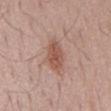{
  "biopsy_status": "not biopsied; imaged during a skin examination",
  "image": {
    "source": "total-body photography crop",
    "field_of_view_mm": 15
  },
  "site": "chest",
  "patient": {
    "sex": "male",
    "age_approx": 65
  },
  "lesion_size": {
    "long_diameter_mm_approx": 5.0
  }
}